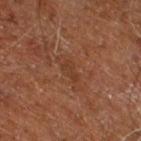| feature | finding |
|---|---|
| notes | total-body-photography surveillance lesion; no biopsy |
| patient | male, aged 58–62 |
| location | the right leg |
| acquisition | ~15 mm tile from a whole-body skin photo |
| size | ~2.5 mm (longest diameter) |